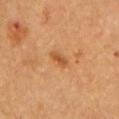The total-body-photography lesion software estimated an eccentricity of roughly 0.85 and a symmetry-axis asymmetry near 0.2. The software also gave border irregularity of about 2 on a 0–10 scale, a within-lesion color-variation index near 1.5/10, and radial color variation of about 0.5. The tile uses cross-polarized illumination. A male subject about 65 years old. About 2.5 mm across. A 15 mm crop from a total-body photograph taken for skin-cancer surveillance. On the left upper arm.